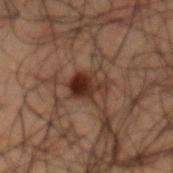Case summary:
* follow-up · total-body-photography surveillance lesion; no biopsy
* body site · the back
* diameter · ≈3.5 mm
* image · total-body-photography crop, ~15 mm field of view
* patient · male, in their 50s
* automated metrics · internal color variation of about 5.5 on a 0–10 scale
* lighting · cross-polarized illumination